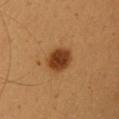This lesion was catalogued during total-body skin photography and was not selected for biopsy. A 15 mm close-up tile from a total-body photography series done for melanoma screening. The patient is a female aged around 25. The lesion-visualizer software estimated a lesion area of about 8 mm², an outline eccentricity of about 0.5 (0 = round, 1 = elongated), and a symmetry-axis asymmetry near 0.1. And it measured a nevus-likeness score of about 100/100 and a lesion-detection confidence of about 100/100. The lesion is on the head or neck.